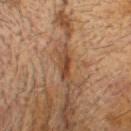biopsy_status: not biopsied; imaged during a skin examination
image:
  source: total-body photography crop
  field_of_view_mm: 15
lighting: cross-polarized
patient:
  sex: male
  age_approx: 75
site: head or neck